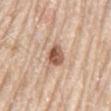Captured during whole-body skin photography for melanoma surveillance; the lesion was not biopsied. The patient is a male in their 80s. A 15 mm close-up tile from a total-body photography series done for melanoma screening. The tile uses white-light illumination. Located on the back.